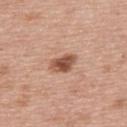Q: Was this lesion biopsied?
A: catalogued during a skin exam; not biopsied
Q: How large is the lesion?
A: about 3.5 mm
Q: What are the patient's age and sex?
A: male, aged 63 to 67
Q: What kind of image is this?
A: total-body-photography crop, ~15 mm field of view
Q: Lesion location?
A: the upper back
Q: How was the tile lit?
A: white-light illumination